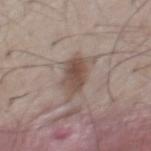Part of a total-body skin-imaging series; this lesion was reviewed on a skin check and was not flagged for biopsy.
Automated image analysis of the tile measured a border-irregularity rating of about 3.5/10, internal color variation of about 4 on a 0–10 scale, and peripheral color asymmetry of about 1.5. The software also gave a classifier nevus-likeness of about 95/100 and a detector confidence of about 100 out of 100 that the crop contains a lesion.
Imaged with white-light lighting.
A male patient in their mid-50s.
This image is a 15 mm lesion crop taken from a total-body photograph.
The lesion's longest dimension is about 5 mm.